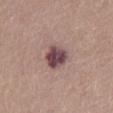Notes:
* illumination — white-light illumination
* patient — female, in their mid- to late 60s
* diameter — ≈3.5 mm
* site — the abdomen
* imaging modality — ~15 mm tile from a whole-body skin photo